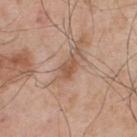No biopsy was performed on this lesion — it was imaged during a full skin examination and was not determined to be concerning.
Measured at roughly 2.5 mm in maximum diameter.
A 15 mm close-up extracted from a 3D total-body photography capture.
A male patient aged around 55.
Imaged with white-light lighting.
The lesion is located on the upper back.